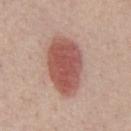biopsy_status: not biopsied; imaged during a skin examination
lighting: white-light
lesion_size:
  long_diameter_mm_approx: 6.5
patient:
  sex: male
  age_approx: 40
image:
  source: total-body photography crop
  field_of_view_mm: 15
site: chest